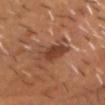Captured during whole-body skin photography for melanoma surveillance; the lesion was not biopsied. The tile uses cross-polarized illumination. The patient is a male aged 53–57. A close-up tile cropped from a whole-body skin photograph, about 15 mm across. On the head or neck.